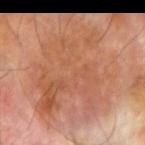biopsy_status: not biopsied; imaged during a skin examination